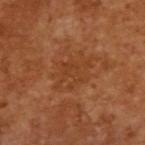| feature | finding |
|---|---|
| biopsy status | total-body-photography surveillance lesion; no biopsy |
| diameter | about 5.5 mm |
| patient | male, aged 63–67 |
| image-analysis metrics | an area of roughly 13 mm², a shape eccentricity near 0.8, and two-axis asymmetry of about 0.3; roughly 5 lightness units darker than nearby skin; a nevus-likeness score of about 0/100 and lesion-presence confidence of about 100/100 |
| acquisition | ~15 mm crop, total-body skin-cancer survey |